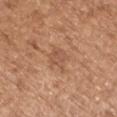Q: Was a biopsy performed?
A: imaged on a skin check; not biopsied
Q: What are the patient's age and sex?
A: female, aged 73–77
Q: Lesion location?
A: the left upper arm
Q: Lesion size?
A: about 3.5 mm
Q: Automated lesion metrics?
A: a footprint of about 6.5 mm²; a classifier nevus-likeness of about 0/100
Q: What is the imaging modality?
A: ~15 mm crop, total-body skin-cancer survey